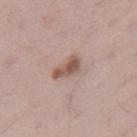Cropped from a whole-body photographic skin survey; the tile spans about 15 mm. From the right thigh. The subject is a male about 40 years old. Longest diameter approximately 3.5 mm. Imaged with white-light lighting.